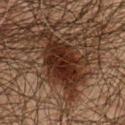Part of a total-body skin-imaging series; this lesion was reviewed on a skin check and was not flagged for biopsy.
A 15 mm close-up tile from a total-body photography series done for melanoma screening.
An algorithmic analysis of the crop reported a lesion area of about 24 mm² and a shape eccentricity near 0.85. The analysis additionally found an average lesion color of about L≈20 a*≈15 b*≈20 (CIELAB), about 11 CIELAB-L* units darker than the surrounding skin, and a normalized border contrast of about 12.5. And it measured a border-irregularity index near 5.5/10, a within-lesion color-variation index near 4/10, and radial color variation of about 1.
On the chest.
The subject is a male aged approximately 60.
Imaged with cross-polarized lighting.